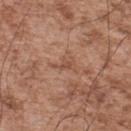No biopsy was performed on this lesion — it was imaged during a full skin examination and was not determined to be concerning. An algorithmic analysis of the crop reported an area of roughly 2.5 mm², a shape eccentricity near 0.85, and a symmetry-axis asymmetry near 0.4. The analysis additionally found an average lesion color of about L≈50 a*≈21 b*≈30 (CIELAB), roughly 7 lightness units darker than nearby skin, and a normalized lesion–skin contrast near 5.5. The software also gave a nevus-likeness score of about 0/100 and lesion-presence confidence of about 90/100. On the upper back. Imaged with white-light lighting. A male patient, aged 53–57. A 15 mm crop from a total-body photograph taken for skin-cancer surveillance.